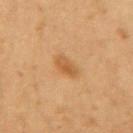workup: imaged on a skin check; not biopsied
patient: female, aged around 20
location: the left forearm
acquisition: total-body-photography crop, ~15 mm field of view